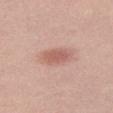workup = catalogued during a skin exam; not biopsied
body site = the right thigh
acquisition = ~15 mm tile from a whole-body skin photo
patient = female, about 20 years old